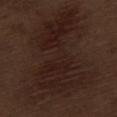Part of a total-body skin-imaging series; this lesion was reviewed on a skin check and was not flagged for biopsy.
About 14 mm across.
The subject is a male in their 70s.
Located on the left thigh.
This is a white-light tile.
The total-body-photography lesion software estimated a mean CIELAB color near L≈20 a*≈15 b*≈19 and a lesion–skin lightness drop of about 5. The analysis additionally found a classifier nevus-likeness of about 15/100 and a detector confidence of about 100 out of 100 that the crop contains a lesion.
Cropped from a whole-body photographic skin survey; the tile spans about 15 mm.